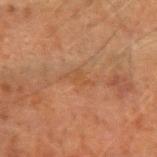Clinical impression: Captured during whole-body skin photography for melanoma surveillance; the lesion was not biopsied. Image and clinical context: The lesion's longest dimension is about 3.5 mm. The lesion is on the left forearm. A male patient in their 60s. The tile uses cross-polarized illumination. A close-up tile cropped from a whole-body skin photograph, about 15 mm across.